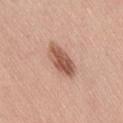The lesion was tiled from a total-body skin photograph and was not biopsied. The subject is a male aged approximately 75. The lesion is on the right thigh. A lesion tile, about 15 mm wide, cut from a 3D total-body photograph.